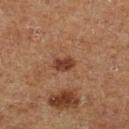Clinical impression:
The lesion was tiled from a total-body skin photograph and was not biopsied.
Clinical summary:
The recorded lesion diameter is about 2.5 mm. Located on the left lower leg. This is a cross-polarized tile. A lesion tile, about 15 mm wide, cut from a 3D total-body photograph. The total-body-photography lesion software estimated an average lesion color of about L≈31 a*≈19 b*≈25 (CIELAB), roughly 9 lightness units darker than nearby skin, and a normalized lesion–skin contrast near 9. It also reported a border-irregularity rating of about 2.5/10, a color-variation rating of about 2/10, and a peripheral color-asymmetry measure near 1. A male patient, roughly 75 years of age.